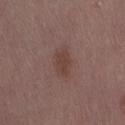  biopsy_status: not biopsied; imaged during a skin examination
  patient:
    sex: female
    age_approx: 55
  site: right thigh
  image:
    source: total-body photography crop
    field_of_view_mm: 15